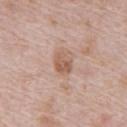Assessment:
Recorded during total-body skin imaging; not selected for excision or biopsy.
Background:
Measured at roughly 2.5 mm in maximum diameter. Automated tile analysis of the lesion measured a lesion area of about 5.5 mm², an eccentricity of roughly 0.6, and a symmetry-axis asymmetry near 0.15. The software also gave a normalized lesion–skin contrast near 7. The software also gave a border-irregularity index near 1.5/10, a color-variation rating of about 4/10, and radial color variation of about 1.5. The analysis additionally found a nevus-likeness score of about 10/100 and lesion-presence confidence of about 100/100. Imaged with white-light lighting. The subject is a male aged approximately 70. The lesion is on the front of the torso. A region of skin cropped from a whole-body photographic capture, roughly 15 mm wide.